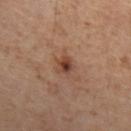This lesion was catalogued during total-body skin photography and was not selected for biopsy.